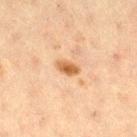The lesion was photographed on a routine skin check and not biopsied; there is no pathology result.
On the right thigh.
Cropped from a total-body skin-imaging series; the visible field is about 15 mm.
A female patient, about 40 years old.